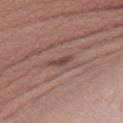No biopsy was performed on this lesion — it was imaged during a full skin examination and was not determined to be concerning.
A 15 mm close-up extracted from a 3D total-body photography capture.
The lesion is on the right upper arm.
A female subject, in their mid-40s.